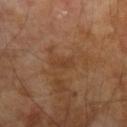Part of a total-body skin-imaging series; this lesion was reviewed on a skin check and was not flagged for biopsy. A male subject in their 70s. A 15 mm close-up extracted from a 3D total-body photography capture. From the left forearm. The lesion-visualizer software estimated an outline eccentricity of about 0.9 (0 = round, 1 = elongated) and a shape-asymmetry score of about 0.4 (0 = symmetric). The software also gave a mean CIELAB color near L≈38 a*≈20 b*≈31, roughly 5 lightness units darker than nearby skin, and a lesion-to-skin contrast of about 5 (normalized; higher = more distinct). The software also gave radial color variation of about 0. The analysis additionally found an automated nevus-likeness rating near 0 out of 100 and a detector confidence of about 100 out of 100 that the crop contains a lesion.